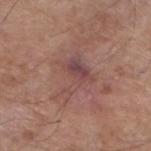No biopsy was performed on this lesion — it was imaged during a full skin examination and was not determined to be concerning. Captured under white-light illumination. The lesion-visualizer software estimated a lesion area of about 10 mm² and a symmetry-axis asymmetry near 0.65. The software also gave an average lesion color of about L≈47 a*≈21 b*≈21 (CIELAB), about 7 CIELAB-L* units darker than the surrounding skin, and a normalized lesion–skin contrast near 6.5. And it measured a border-irregularity index near 8/10, internal color variation of about 5 on a 0–10 scale, and radial color variation of about 1.5. The analysis additionally found a lesion-detection confidence of about 95/100. The lesion is on the leg. The subject is a male approximately 80 years of age. A roughly 15 mm field-of-view crop from a total-body skin photograph.